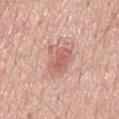<case>
  <biopsy_status>not biopsied; imaged during a skin examination</biopsy_status>
  <site>mid back</site>
  <image>
    <source>total-body photography crop</source>
    <field_of_view_mm>15</field_of_view_mm>
  </image>
  <patient>
    <sex>male</sex>
    <age_approx>65</age_approx>
  </patient>
</case>